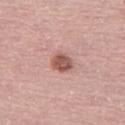workup=no biopsy performed (imaged during a skin exam) | patient=female, approximately 60 years of age | size=about 3 mm | tile lighting=white-light illumination | image=~15 mm tile from a whole-body skin photo | image-analysis metrics=a border-irregularity rating of about 1.5/10, a color-variation rating of about 3/10, and peripheral color asymmetry of about 1 | site=the right thigh.